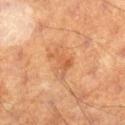Findings:
– follow-up — no biopsy performed (imaged during a skin exam)
– tile lighting — cross-polarized illumination
– patient — male, aged around 60
– imaging modality — 15 mm crop, total-body photography
– lesion size — about 3.5 mm
– body site — the right lower leg